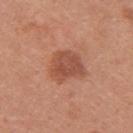follow-up: catalogued during a skin exam; not biopsied
location: the right upper arm
automated metrics: a footprint of about 11 mm², an eccentricity of roughly 0.65, and a shape-asymmetry score of about 0.3 (0 = symmetric); a border-irregularity index near 3/10, a color-variation rating of about 3.5/10, and a peripheral color-asymmetry measure near 1; a detector confidence of about 100 out of 100 that the crop contains a lesion
subject: female, aged 33 to 37
lesion size: ≈4.5 mm
imaging modality: ~15 mm tile from a whole-body skin photo